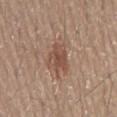Assessment: No biopsy was performed on this lesion — it was imaged during a full skin examination and was not determined to be concerning. Background: This image is a 15 mm lesion crop taken from a total-body photograph. On the lower back. A male subject in their 20s.